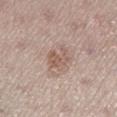Notes:
– biopsy status · no biopsy performed (imaged during a skin exam)
– patient · female, roughly 50 years of age
– image source · ~15 mm crop, total-body skin-cancer survey
– lighting · white-light illumination
– site · the leg
– lesion diameter · ≈3 mm